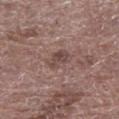  biopsy_status: not biopsied; imaged during a skin examination
  lighting: white-light
  patient:
    sex: male
    age_approx: 70
  lesion_size:
    long_diameter_mm_approx: 3.0
  site: left lower leg
  automated_metrics:
    eccentricity: 0.75
    vs_skin_darker_L: 8.0
    vs_skin_contrast_norm: 6.5
    border_irregularity_0_10: 2.5
    color_variation_0_10: 3.5
    nevus_likeness_0_100: 0
    lesion_detection_confidence_0_100: 100
  image:
    source: total-body photography crop
    field_of_view_mm: 15